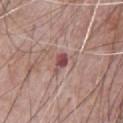Findings:
– notes · total-body-photography surveillance lesion; no biopsy
– lighting · white-light illumination
– subject · male, aged 68 to 72
– image source · ~15 mm crop, total-body skin-cancer survey
– automated lesion analysis · an area of roughly 3 mm², a shape eccentricity near 0.8, and two-axis asymmetry of about 0.4
– lesion size · about 2.5 mm
– body site · the chest